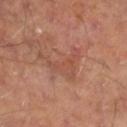<tbp_lesion>
<biopsy_status>not biopsied; imaged during a skin examination</biopsy_status>
<lesion_size>
  <long_diameter_mm_approx>5.5</long_diameter_mm_approx>
</lesion_size>
<automated_metrics>
  <border_irregularity_0_10>9.0</border_irregularity_0_10>
  <color_variation_0_10>3.0</color_variation_0_10>
  <peripheral_color_asymmetry>1.0</peripheral_color_asymmetry>
</automated_metrics>
<image>
  <source>total-body photography crop</source>
  <field_of_view_mm>15</field_of_view_mm>
</image>
<site>right lower leg</site>
<patient>
  <sex>male</sex>
  <age_approx>65</age_approx>
</patient>
<lighting>cross-polarized</lighting>
</tbp_lesion>